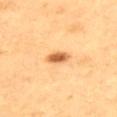| field | value |
|---|---|
| follow-up | total-body-photography surveillance lesion; no biopsy |
| body site | the upper back |
| illumination | cross-polarized |
| patient | male, aged 53 to 57 |
| acquisition | total-body-photography crop, ~15 mm field of view |
| size | ~3 mm (longest diameter) |
| TBP lesion metrics | an automated nevus-likeness rating near 100 out of 100 and lesion-presence confidence of about 100/100 |